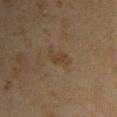This lesion was catalogued during total-body skin photography and was not selected for biopsy. The total-body-photography lesion software estimated a border-irregularity index near 4.5/10 and internal color variation of about 0.5 on a 0–10 scale. The software also gave a classifier nevus-likeness of about 0/100 and a detector confidence of about 100 out of 100 that the crop contains a lesion. A region of skin cropped from a whole-body photographic capture, roughly 15 mm wide. A male patient, roughly 45 years of age. On the arm. Imaged with cross-polarized lighting. The lesion's longest dimension is about 3.5 mm.